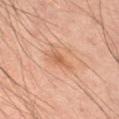The lesion's longest dimension is about 2.5 mm. Automated tile analysis of the lesion measured border irregularity of about 3.5 on a 0–10 scale. The software also gave a classifier nevus-likeness of about 0/100. A 15 mm crop from a total-body photograph taken for skin-cancer surveillance. The tile uses cross-polarized illumination. A male patient roughly 45 years of age. On the right upper arm.